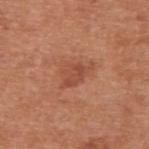biopsy_status: not biopsied; imaged during a skin examination
site: upper back
patient:
  sex: male
  age_approx: 55
lesion_size:
  long_diameter_mm_approx: 3.5
automated_metrics:
  area_mm2_approx: 5.0
  shape_asymmetry: 0.25
  border_irregularity_0_10: 3.5
  color_variation_0_10: 2.0
lighting: white-light
image:
  source: total-body photography crop
  field_of_view_mm: 15